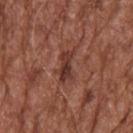Q: Was a biopsy performed?
A: imaged on a skin check; not biopsied
Q: Who is the patient?
A: male, aged 73–77
Q: What is the imaging modality?
A: total-body-photography crop, ~15 mm field of view
Q: What is the anatomic site?
A: the upper back